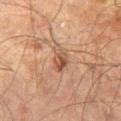Recorded during total-body skin imaging; not selected for excision or biopsy.
The tile uses cross-polarized illumination.
A roughly 15 mm field-of-view crop from a total-body skin photograph.
Longest diameter approximately 3 mm.
An algorithmic analysis of the crop reported a mean CIELAB color near L≈43 a*≈19 b*≈28, about 9 CIELAB-L* units darker than the surrounding skin, and a normalized lesion–skin contrast near 7.
A male subject aged approximately 70.
The lesion is on the left thigh.